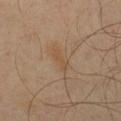| key | value |
|---|---|
| lesion size | ~2.5 mm (longest diameter) |
| location | the chest |
| tile lighting | cross-polarized |
| automated lesion analysis | about 5 CIELAB-L* units darker than the surrounding skin and a lesion-to-skin contrast of about 5.5 (normalized; higher = more distinct); a border-irregularity index near 5/10 |
| subject | male, aged around 40 |
| image | ~15 mm tile from a whole-body skin photo |